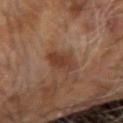Q: Was this lesion biopsied?
A: catalogued during a skin exam; not biopsied
Q: What is the lesion's diameter?
A: ≈4 mm
Q: What did automated image analysis measure?
A: an area of roughly 7 mm² and an outline eccentricity of about 0.85 (0 = round, 1 = elongated); a mean CIELAB color near L≈37 a*≈20 b*≈28, about 9 CIELAB-L* units darker than the surrounding skin, and a normalized lesion–skin contrast near 7.5; a classifier nevus-likeness of about 35/100 and a lesion-detection confidence of about 100/100
Q: Where on the body is the lesion?
A: the right arm
Q: Patient demographics?
A: male, aged 58–62
Q: What is the imaging modality?
A: ~15 mm crop, total-body skin-cancer survey
Q: What lighting was used for the tile?
A: cross-polarized